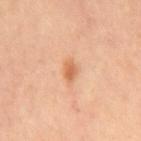Recorded during total-body skin imaging; not selected for excision or biopsy. This image is a 15 mm lesion crop taken from a total-body photograph. About 2.5 mm across. A male patient, approximately 60 years of age. This is a cross-polarized tile. On the mid back. Automated image analysis of the tile measured a lesion–skin lightness drop of about 10 and a normalized lesion–skin contrast near 7. The analysis additionally found border irregularity of about 3 on a 0–10 scale, a within-lesion color-variation index near 2/10, and a peripheral color-asymmetry measure near 0.5. The analysis additionally found an automated nevus-likeness rating near 90 out of 100 and a detector confidence of about 100 out of 100 that the crop contains a lesion.